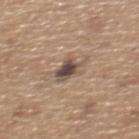Captured during whole-body skin photography for melanoma surveillance; the lesion was not biopsied. A male subject aged around 65. The lesion-visualizer software estimated about 13 CIELAB-L* units darker than the surrounding skin and a normalized border contrast of about 10. It also reported border irregularity of about 5 on a 0–10 scale, a within-lesion color-variation index near 6/10, and a peripheral color-asymmetry measure near 2. The software also gave a classifier nevus-likeness of about 65/100 and a detector confidence of about 100 out of 100 that the crop contains a lesion. A region of skin cropped from a whole-body photographic capture, roughly 15 mm wide. The lesion is on the upper back. The tile uses white-light illumination.